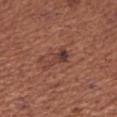Findings:
- workup: imaged on a skin check; not biopsied
- image: total-body-photography crop, ~15 mm field of view
- diameter: about 4 mm
- tile lighting: white-light
- subject: female, aged 48 to 52
- body site: the right upper arm